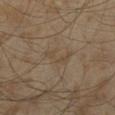Clinical impression: Recorded during total-body skin imaging; not selected for excision or biopsy. Acquisition and patient details: The lesion is on the left thigh. This is a cross-polarized tile. This image is a 15 mm lesion crop taken from a total-body photograph. The lesion-visualizer software estimated an area of roughly 4 mm² and a shape eccentricity near 0.9. The software also gave roughly 5 lightness units darker than nearby skin and a normalized lesion–skin contrast near 4.5. And it measured border irregularity of about 8 on a 0–10 scale, internal color variation of about 0 on a 0–10 scale, and a peripheral color-asymmetry measure near 0. It also reported a classifier nevus-likeness of about 0/100 and a detector confidence of about 95 out of 100 that the crop contains a lesion. A male patient, roughly 60 years of age. Approximately 4 mm at its widest.